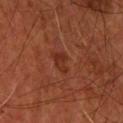workup: catalogued during a skin exam; not biopsied
subject: male, aged 53–57
TBP lesion metrics: a footprint of about 3.5 mm², an eccentricity of roughly 0.8, and a shape-asymmetry score of about 0.4 (0 = symmetric); a lesion–skin lightness drop of about 6 and a normalized lesion–skin contrast near 6.5
lighting: cross-polarized
acquisition: total-body-photography crop, ~15 mm field of view
diameter: ≈2.5 mm
anatomic site: the upper back